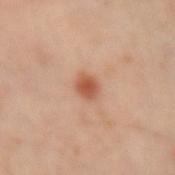Assessment: Captured during whole-body skin photography for melanoma surveillance; the lesion was not biopsied. Image and clinical context: An algorithmic analysis of the crop reported a footprint of about 4 mm², an eccentricity of roughly 0.55, and a symmetry-axis asymmetry near 0.2. The analysis additionally found a lesion color around L≈55 a*≈26 b*≈34 in CIELAB and a lesion–skin lightness drop of about 12. The lesion's longest dimension is about 2.5 mm. The lesion is on the left forearm. A male subject aged 53 to 57. This is a cross-polarized tile. A 15 mm close-up tile from a total-body photography series done for melanoma screening.